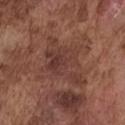Recorded during total-body skin imaging; not selected for excision or biopsy.
The tile uses white-light illumination.
Automated tile analysis of the lesion measured a lesion–skin lightness drop of about 7 and a normalized lesion–skin contrast near 6.5. And it measured a border-irregularity rating of about 5.5/10, a color-variation rating of about 4.5/10, and radial color variation of about 1.5. It also reported an automated nevus-likeness rating near 0 out of 100 and lesion-presence confidence of about 90/100.
A 15 mm close-up tile from a total-body photography series done for melanoma screening.
A male subject aged 73–77.
The lesion is on the front of the torso.